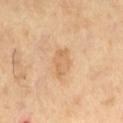The subject is a male about 65 years old.
A 15 mm close-up tile from a total-body photography series done for melanoma screening.